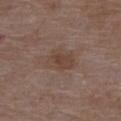No biopsy was performed on this lesion — it was imaged during a full skin examination and was not determined to be concerning. A 15 mm close-up tile from a total-body photography series done for melanoma screening. A female patient roughly 70 years of age. An algorithmic analysis of the crop reported a mean CIELAB color near L≈42 a*≈16 b*≈24 and roughly 6 lightness units darker than nearby skin. From the upper back. The lesion's longest dimension is about 3.5 mm.